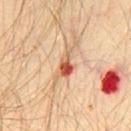Q: Is there a histopathology result?
A: imaged on a skin check; not biopsied
Q: What lighting was used for the tile?
A: cross-polarized
Q: Who is the patient?
A: male, aged around 30
Q: Automated lesion metrics?
A: an area of roughly 6 mm², a shape eccentricity near 0.9, and a symmetry-axis asymmetry near 0.6; about 14 CIELAB-L* units darker than the surrounding skin and a normalized border contrast of about 9; a border-irregularity index near 7/10 and a color-variation rating of about 10/10; an automated nevus-likeness rating near 0 out of 100 and a lesion-detection confidence of about 100/100
Q: Where on the body is the lesion?
A: the front of the torso
Q: What is the lesion's diameter?
A: ~4.5 mm (longest diameter)
Q: What is the imaging modality?
A: 15 mm crop, total-body photography